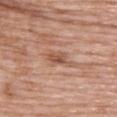This lesion was catalogued during total-body skin photography and was not selected for biopsy.
A 15 mm crop from a total-body photograph taken for skin-cancer surveillance.
From the upper back.
A female patient, aged 68–72.
Imaged with white-light lighting.